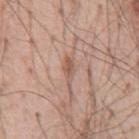No biopsy was performed on this lesion — it was imaged during a full skin examination and was not determined to be concerning. The lesion-visualizer software estimated border irregularity of about 3.5 on a 0–10 scale and internal color variation of about 0.5 on a 0–10 scale. Imaged with white-light lighting. Measured at roughly 2.5 mm in maximum diameter. A male subject in their mid- to late 50s. This image is a 15 mm lesion crop taken from a total-body photograph. Located on the abdomen.